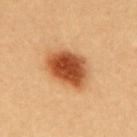Clinical summary:
The recorded lesion diameter is about 5.5 mm. The lesion is located on the back. A lesion tile, about 15 mm wide, cut from a 3D total-body photograph. The subject is a female in their mid-20s. Automated image analysis of the tile measured a lesion area of about 17 mm², an outline eccentricity of about 0.7 (0 = round, 1 = elongated), and a shape-asymmetry score of about 0.15 (0 = symmetric). It also reported a lesion-detection confidence of about 100/100.